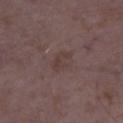- notes · total-body-photography surveillance lesion; no biopsy
- anatomic site · the leg
- TBP lesion metrics · a mean CIELAB color near L≈38 a*≈15 b*≈18, a lesion–skin lightness drop of about 5, and a normalized lesion–skin contrast near 5; border irregularity of about 3 on a 0–10 scale and radial color variation of about 1; a classifier nevus-likeness of about 0/100 and lesion-presence confidence of about 100/100
- diameter · ≈3 mm
- illumination · white-light illumination
- image source · ~15 mm crop, total-body skin-cancer survey
- subject · female, about 35 years old